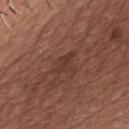No biopsy was performed on this lesion — it was imaged during a full skin examination and was not determined to be concerning.
A region of skin cropped from a whole-body photographic capture, roughly 15 mm wide.
On the chest.
The subject is a male in their mid- to late 70s.
This is a white-light tile.